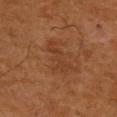Assessment:
Imaged during a routine full-body skin examination; the lesion was not biopsied and no histopathology is available.
Image and clinical context:
The lesion is located on the back. The recorded lesion diameter is about 4 mm. The patient is a male about 60 years old. This is a cross-polarized tile. A close-up tile cropped from a whole-body skin photograph, about 15 mm across. Automated image analysis of the tile measured roughly 5 lightness units darker than nearby skin and a lesion-to-skin contrast of about 4.5 (normalized; higher = more distinct). The analysis additionally found a border-irregularity rating of about 8.5/10 and a within-lesion color-variation index near 0/10. The analysis additionally found a classifier nevus-likeness of about 5/100.